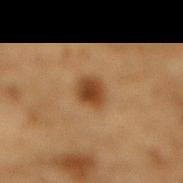The lesion was tiled from a total-body skin photograph and was not biopsied. Cropped from a total-body skin-imaging series; the visible field is about 15 mm. A male patient aged 83 or older. On the mid back. Captured under cross-polarized illumination. About 2.5 mm across.